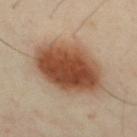This lesion was catalogued during total-body skin photography and was not selected for biopsy. A 15 mm close-up tile from a total-body photography series done for melanoma screening. The patient is a male aged 38 to 42. The lesion is located on the chest.